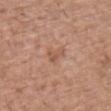* size — ~2.5 mm (longest diameter)
* subject — male, about 70 years old
* image-analysis metrics — an area of roughly 4 mm² and a shape eccentricity near 0.65; a lesion color around L≈55 a*≈20 b*≈30 in CIELAB and roughly 7 lightness units darker than nearby skin; a border-irregularity index near 5/10; a classifier nevus-likeness of about 0/100 and a lesion-detection confidence of about 100/100
* image — 15 mm crop, total-body photography
* body site — the front of the torso